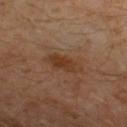Recorded during total-body skin imaging; not selected for excision or biopsy. The subject is a male about 30 years old. A 15 mm close-up extracted from a 3D total-body photography capture. Automated image analysis of the tile measured an average lesion color of about L≈36 a*≈19 b*≈29 (CIELAB) and a normalized border contrast of about 7.5. It also reported border irregularity of about 3 on a 0–10 scale, a within-lesion color-variation index near 3.5/10, and peripheral color asymmetry of about 1. The analysis additionally found a nevus-likeness score of about 10/100 and a lesion-detection confidence of about 100/100. The recorded lesion diameter is about 5 mm. The lesion is located on the leg. Imaged with cross-polarized lighting.